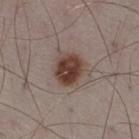Q: Was a biopsy performed?
A: no biopsy performed (imaged during a skin exam)
Q: Patient demographics?
A: male, approximately 50 years of age
Q: Lesion location?
A: the right thigh
Q: What kind of image is this?
A: 15 mm crop, total-body photography